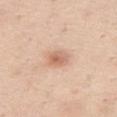| key | value |
|---|---|
| notes | total-body-photography surveillance lesion; no biopsy |
| subject | female, in their mid- to late 50s |
| image | 15 mm crop, total-body photography |
| location | the right thigh |